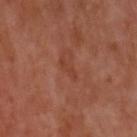Captured during whole-body skin photography for melanoma surveillance; the lesion was not biopsied. From the upper back. A male subject, in their mid- to late 50s. A 15 mm close-up tile from a total-body photography series done for melanoma screening.